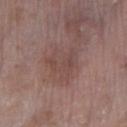No biopsy was performed on this lesion — it was imaged during a full skin examination and was not determined to be concerning. A roughly 15 mm field-of-view crop from a total-body skin photograph. A female subject, approximately 70 years of age. Measured at roughly 4 mm in maximum diameter. The lesion is on the right lower leg. An algorithmic analysis of the crop reported a lesion area of about 9.5 mm², a shape eccentricity near 0.5, and a symmetry-axis asymmetry near 0.4. The software also gave a lesion–skin lightness drop of about 6 and a normalized lesion–skin contrast near 5. It also reported a border-irregularity index near 5.5/10, a color-variation rating of about 2.5/10, and radial color variation of about 1. It also reported a classifier nevus-likeness of about 0/100 and a lesion-detection confidence of about 100/100.